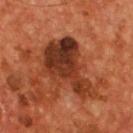The patient is a male roughly 55 years of age. From the chest. A 15 mm close-up extracted from a 3D total-body photography capture.